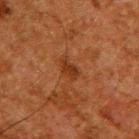No biopsy was performed on this lesion — it was imaged during a full skin examination and was not determined to be concerning. Approximately 3 mm at its widest. The subject is a male roughly 60 years of age. The lesion is located on the upper back. A region of skin cropped from a whole-body photographic capture, roughly 15 mm wide.